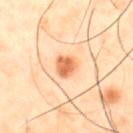Impression: The lesion was photographed on a routine skin check and not biopsied; there is no pathology result. Acquisition and patient details: A 15 mm close-up tile from a total-body photography series done for melanoma screening. From the abdomen. Measured at roughly 2.5 mm in maximum diameter. The tile uses cross-polarized illumination. A male patient, in their 50s.